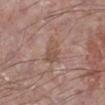Imaged during a routine full-body skin examination; the lesion was not biopsied and no histopathology is available. The subject is a male approximately 65 years of age. The recorded lesion diameter is about 3.5 mm. Imaged with white-light lighting. A 15 mm crop from a total-body photograph taken for skin-cancer surveillance. On the left lower leg. The lesion-visualizer software estimated border irregularity of about 4.5 on a 0–10 scale, a within-lesion color-variation index near 1.5/10, and peripheral color asymmetry of about 0.5. The software also gave lesion-presence confidence of about 100/100.